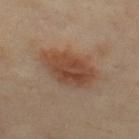Clinical impression:
Recorded during total-body skin imaging; not selected for excision or biopsy.
Background:
The recorded lesion diameter is about 6 mm. From the upper back. A male patient, aged around 45. Cropped from a total-body skin-imaging series; the visible field is about 15 mm.